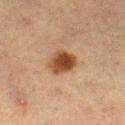  biopsy_status: not biopsied; imaged during a skin examination
  image:
    source: total-body photography crop
    field_of_view_mm: 15
  lesion_size:
    long_diameter_mm_approx: 3.5
  automated_metrics:
    cielab_L: 38
    cielab_a: 18
    cielab_b: 29
    vs_skin_contrast_norm: 11.0
    color_variation_0_10: 5.0
    peripheral_color_asymmetry: 1.5
  patient:
    sex: female
    age_approx: 55
  site: leg